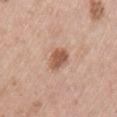workup=no biopsy performed (imaged during a skin exam); lighting=white-light; image-analysis metrics=an average lesion color of about L≈56 a*≈22 b*≈30 (CIELAB) and a lesion–skin lightness drop of about 12; lesion size=about 3 mm; image source=~15 mm tile from a whole-body skin photo; subject=female, about 50 years old; location=the left upper arm.